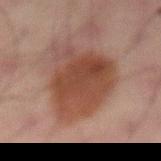Impression:
Recorded during total-body skin imaging; not selected for excision or biopsy.
Acquisition and patient details:
A 15 mm crop from a total-body photograph taken for skin-cancer surveillance. This is a cross-polarized tile. The total-body-photography lesion software estimated a lesion color around L≈36 a*≈18 b*≈23 in CIELAB, a lesion–skin lightness drop of about 9, and a normalized border contrast of about 9. Measured at roughly 8 mm in maximum diameter. The lesion is located on the lower back. The subject is a male aged around 65.